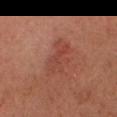Recorded during total-body skin imaging; not selected for excision or biopsy. Measured at roughly 4.5 mm in maximum diameter. The subject is a male in their mid-60s. Imaged with cross-polarized lighting. On the head or neck. Automated image analysis of the tile measured an average lesion color of about L≈42 a*≈25 b*≈27 (CIELAB), about 6 CIELAB-L* units darker than the surrounding skin, and a normalized border contrast of about 5. Cropped from a total-body skin-imaging series; the visible field is about 15 mm.